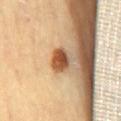No biopsy was performed on this lesion — it was imaged during a full skin examination and was not determined to be concerning.
A roughly 15 mm field-of-view crop from a total-body skin photograph.
A female subject approximately 70 years of age.
Imaged with cross-polarized lighting.
On the mid back.